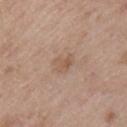Clinical impression:
Captured during whole-body skin photography for melanoma surveillance; the lesion was not biopsied.
Background:
A region of skin cropped from a whole-body photographic capture, roughly 15 mm wide. The patient is a male aged 48 to 52. The lesion is on the left upper arm.